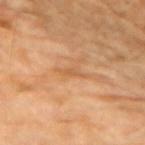Recorded during total-body skin imaging; not selected for excision or biopsy.
Measured at roughly 2.5 mm in maximum diameter.
A female patient, aged approximately 70.
From the left upper arm.
A 15 mm crop from a total-body photograph taken for skin-cancer surveillance.
The tile uses cross-polarized illumination.